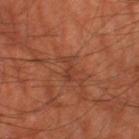workup: no biopsy performed (imaged during a skin exam)
site: the right thigh
imaging modality: ~15 mm tile from a whole-body skin photo
patient: male, aged around 65
size: ~2.5 mm (longest diameter)
lighting: cross-polarized
image-analysis metrics: border irregularity of about 5.5 on a 0–10 scale, internal color variation of about 0 on a 0–10 scale, and a peripheral color-asymmetry measure near 0